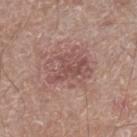The lesion was tiled from a total-body skin photograph and was not biopsied. On the right lower leg. The lesion-visualizer software estimated a footprint of about 13 mm². And it measured a mean CIELAB color near L≈51 a*≈21 b*≈22. It also reported a border-irregularity rating of about 4.5/10, internal color variation of about 5 on a 0–10 scale, and a peripheral color-asymmetry measure near 2. It also reported a nevus-likeness score of about 0/100. A region of skin cropped from a whole-body photographic capture, roughly 15 mm wide. The subject is a male aged approximately 60.